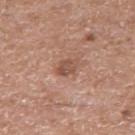workup: catalogued during a skin exam; not biopsied
body site: the right upper arm
patient: male, aged 53 to 57
image: 15 mm crop, total-body photography
tile lighting: white-light illumination